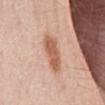Findings:
- biopsy status · imaged on a skin check; not biopsied
- subject · male, about 60 years old
- image source · ~15 mm tile from a whole-body skin photo
- lighting · white-light illumination
- site · the abdomen
- lesion size · ≈5 mm